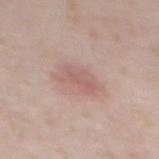Findings:
* workup — no biopsy performed (imaged during a skin exam)
* illumination — white-light
* TBP lesion metrics — a lesion color around L≈59 a*≈21 b*≈22 in CIELAB and a normalized lesion–skin contrast near 5
* imaging modality — total-body-photography crop, ~15 mm field of view
* body site — the mid back
* subject — male, roughly 40 years of age